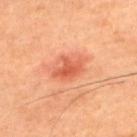Q: Was this lesion biopsied?
A: no biopsy performed (imaged during a skin exam)
Q: Illumination type?
A: cross-polarized
Q: What are the patient's age and sex?
A: male, aged 43 to 47
Q: Lesion location?
A: the upper back
Q: What is the imaging modality?
A: ~15 mm tile from a whole-body skin photo